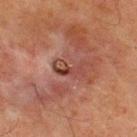{"biopsy_status": "not biopsied; imaged during a skin examination", "patient": {"sex": "male", "age_approx": 60}, "lighting": "cross-polarized", "image": {"source": "total-body photography crop", "field_of_view_mm": 15}, "lesion_size": {"long_diameter_mm_approx": 3.5}, "site": "upper back", "automated_metrics": {"area_mm2_approx": 5.0, "eccentricity": 0.9, "cielab_L": 33, "cielab_a": 20, "cielab_b": 22, "vs_skin_contrast_norm": 7.0, "nevus_likeness_0_100": 0, "lesion_detection_confidence_0_100": 90}}